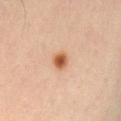Q: Is there a histopathology result?
A: no biopsy performed (imaged during a skin exam)
Q: Illumination type?
A: cross-polarized illumination
Q: What kind of image is this?
A: ~15 mm tile from a whole-body skin photo
Q: Where on the body is the lesion?
A: the left thigh
Q: Who is the patient?
A: male, approximately 65 years of age
Q: Lesion size?
A: ~2.5 mm (longest diameter)